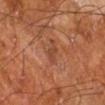biopsy status=no biopsy performed (imaged during a skin exam) | diameter=~3.5 mm (longest diameter) | acquisition=~15 mm crop, total-body skin-cancer survey | patient=male, approximately 65 years of age | lighting=cross-polarized illumination | image-analysis metrics=roughly 7 lightness units darker than nearby skin and a normalized border contrast of about 5.5; internal color variation of about 1.5 on a 0–10 scale; an automated nevus-likeness rating near 0 out of 100 and a detector confidence of about 100 out of 100 that the crop contains a lesion | site=the left lower leg.